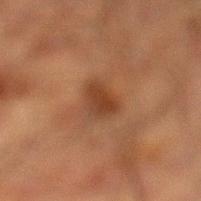Impression:
This lesion was catalogued during total-body skin photography and was not selected for biopsy.
Acquisition and patient details:
A 15 mm close-up extracted from a 3D total-body photography capture. Measured at roughly 3.5 mm in maximum diameter. The lesion is located on the left lower leg. The patient is a male aged around 50.